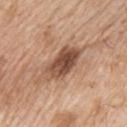Q: What is the imaging modality?
A: total-body-photography crop, ~15 mm field of view
Q: What are the patient's age and sex?
A: male, aged around 65
Q: Where on the body is the lesion?
A: the left upper arm